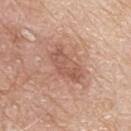biopsy status = total-body-photography surveillance lesion; no biopsy
location = the upper back
size = about 5 mm
patient = male, about 80 years old
image source = 15 mm crop, total-body photography
tile lighting = white-light